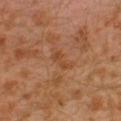Impression:
No biopsy was performed on this lesion — it was imaged during a full skin examination and was not determined to be concerning.
Clinical summary:
On the left leg. The subject is a male aged 28 to 32. A 15 mm close-up tile from a total-body photography series done for melanoma screening.